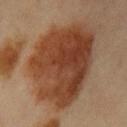The lesion was tiled from a total-body skin photograph and was not biopsied. Measured at roughly 9.5 mm in maximum diameter. The lesion is on the left upper arm. A lesion tile, about 15 mm wide, cut from a 3D total-body photograph. A female patient, approximately 45 years of age.